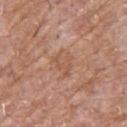Findings:
* workup — no biopsy performed (imaged during a skin exam)